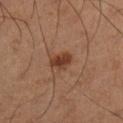<tbp_lesion>
<biopsy_status>not biopsied; imaged during a skin examination</biopsy_status>
<site>left lower leg</site>
<image>
  <source>total-body photography crop</source>
  <field_of_view_mm>15</field_of_view_mm>
</image>
<patient>
  <sex>male</sex>
  <age_approx>60</age_approx>
</patient>
<lighting>cross-polarized</lighting>
<lesion_size>
  <long_diameter_mm_approx>2.5</long_diameter_mm_approx>
</lesion_size>
</tbp_lesion>